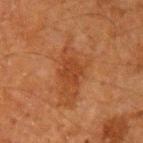Assessment: This lesion was catalogued during total-body skin photography and was not selected for biopsy. Image and clinical context: Captured under cross-polarized illumination. A lesion tile, about 15 mm wide, cut from a 3D total-body photograph. Automated tile analysis of the lesion measured a footprint of about 5 mm², a shape eccentricity near 0.65, and two-axis asymmetry of about 0.15. And it measured about 6 CIELAB-L* units darker than the surrounding skin. It also reported border irregularity of about 2 on a 0–10 scale, internal color variation of about 1.5 on a 0–10 scale, and radial color variation of about 0.5. The analysis additionally found a classifier nevus-likeness of about 0/100 and a detector confidence of about 100 out of 100 that the crop contains a lesion. Longest diameter approximately 2.5 mm. On the arm. A male patient, approximately 60 years of age.